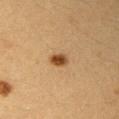Impression: Imaged during a routine full-body skin examination; the lesion was not biopsied and no histopathology is available. Background: From the right upper arm. The subject is a female aged around 30. The recorded lesion diameter is about 2.5 mm. The tile uses cross-polarized illumination. A roughly 15 mm field-of-view crop from a total-body skin photograph.